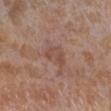Clinical impression: The lesion was photographed on a routine skin check and not biopsied; there is no pathology result. Clinical summary: Measured at roughly 3 mm in maximum diameter. Cropped from a total-body skin-imaging series; the visible field is about 15 mm. Automated tile analysis of the lesion measured an area of roughly 4.5 mm², an outline eccentricity of about 0.7 (0 = round, 1 = elongated), and a shape-asymmetry score of about 0.45 (0 = symmetric). It also reported an average lesion color of about L≈48 a*≈20 b*≈27 (CIELAB), a lesion–skin lightness drop of about 6, and a normalized lesion–skin contrast near 5.5. The software also gave a border-irregularity index near 4.5/10. Located on the right lower leg. A female patient, approximately 55 years of age.